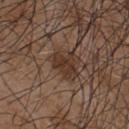Assessment: Captured during whole-body skin photography for melanoma surveillance; the lesion was not biopsied. Background: A roughly 15 mm field-of-view crop from a total-body skin photograph. The tile uses white-light illumination. A male subject, in their mid- to late 50s. The lesion is on the chest.